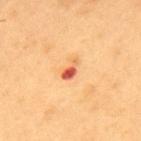follow-up = no biopsy performed (imaged during a skin exam); lesion diameter = about 2.5 mm; location = the back; lighting = cross-polarized illumination; subject = male, aged 53 to 57; acquisition = total-body-photography crop, ~15 mm field of view.